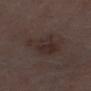A male patient approximately 70 years of age. Cropped from a whole-body photographic skin survey; the tile spans about 15 mm. Imaged with white-light lighting. Located on the left lower leg. The lesion-visualizer software estimated an area of roughly 14 mm², an eccentricity of roughly 0.85, and a shape-asymmetry score of about 0.35 (0 = symmetric). The analysis additionally found an average lesion color of about L≈28 a*≈13 b*≈16 (CIELAB), a lesion–skin lightness drop of about 6, and a lesion-to-skin contrast of about 6.5 (normalized; higher = more distinct). It also reported an automated nevus-likeness rating near 10 out of 100.